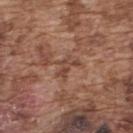  biopsy_status: not biopsied; imaged during a skin examination
  image:
    source: total-body photography crop
    field_of_view_mm: 15
  lighting: white-light
  patient:
    sex: male
    age_approx: 75
  site: upper back